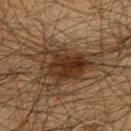The lesion was photographed on a routine skin check and not biopsied; there is no pathology result.
A close-up tile cropped from a whole-body skin photograph, about 15 mm across.
About 6 mm across.
The lesion is located on the abdomen.
The patient is a male approximately 60 years of age.
The tile uses cross-polarized illumination.